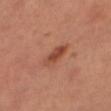Findings:
• notes: catalogued during a skin exam; not biopsied
• imaging modality: total-body-photography crop, ~15 mm field of view
• size: ≈4 mm
• image-analysis metrics: a lesion color around L≈46 a*≈26 b*≈32 in CIELAB, about 10 CIELAB-L* units darker than the surrounding skin, and a lesion-to-skin contrast of about 7.5 (normalized; higher = more distinct); radial color variation of about 1; a nevus-likeness score of about 95/100
• subject: female
• site: the right thigh
• lighting: cross-polarized